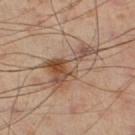Recorded during total-body skin imaging; not selected for excision or biopsy.
Located on the right lower leg.
The recorded lesion diameter is about 7 mm.
A male subject in their mid-50s.
A close-up tile cropped from a whole-body skin photograph, about 15 mm across.